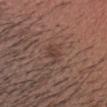Clinical impression:
Captured during whole-body skin photography for melanoma surveillance; the lesion was not biopsied.
Background:
A male patient aged approximately 40. This image is a 15 mm lesion crop taken from a total-body photograph. From the head or neck. An algorithmic analysis of the crop reported a footprint of about 3 mm² and an eccentricity of roughly 0.8. The analysis additionally found a mean CIELAB color near L≈40 a*≈18 b*≈23 and a normalized lesion–skin contrast near 5.5. The analysis additionally found a border-irregularity index near 3/10 and peripheral color asymmetry of about 0.5. And it measured a detector confidence of about 100 out of 100 that the crop contains a lesion. Imaged with white-light lighting.